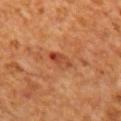Q: What is the imaging modality?
A: ~15 mm tile from a whole-body skin photo
Q: What lighting was used for the tile?
A: cross-polarized
Q: What is the anatomic site?
A: the back
Q: What did automated image analysis measure?
A: border irregularity of about 3 on a 0–10 scale and radial color variation of about 2.5; an automated nevus-likeness rating near 0 out of 100 and a detector confidence of about 100 out of 100 that the crop contains a lesion
Q: What are the patient's age and sex?
A: male, aged around 65
Q: Lesion size?
A: about 3.5 mm